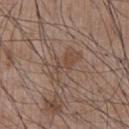No biopsy was performed on this lesion — it was imaged during a full skin examination and was not determined to be concerning. An algorithmic analysis of the crop reported a lesion area of about 9.5 mm², an eccentricity of roughly 0.85, and a symmetry-axis asymmetry near 0.35. It also reported a mean CIELAB color near L≈47 a*≈16 b*≈25, a lesion–skin lightness drop of about 6, and a lesion-to-skin contrast of about 5.5 (normalized; higher = more distinct). A close-up tile cropped from a whole-body skin photograph, about 15 mm across. The lesion is on the chest. Captured under white-light illumination. The patient is a male aged around 55.